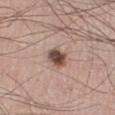  biopsy_status: not biopsied; imaged during a skin examination
  patient:
    sex: male
    age_approx: 35
  lighting: white-light
  site: right lower leg
  image:
    source: total-body photography crop
    field_of_view_mm: 15
  lesion_size:
    long_diameter_mm_approx: 3.0